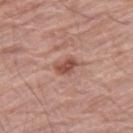Impression:
Recorded during total-body skin imaging; not selected for excision or biopsy.
Image and clinical context:
Automated image analysis of the tile measured a lesion–skin lightness drop of about 12 and a lesion-to-skin contrast of about 8 (normalized; higher = more distinct). The analysis additionally found a nevus-likeness score of about 80/100. This image is a 15 mm lesion crop taken from a total-body photograph. Approximately 3 mm at its widest. A male patient, aged approximately 80. The tile uses white-light illumination. Located on the right thigh.